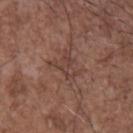Case summary:
* workup — total-body-photography surveillance lesion; no biopsy
* image — ~15 mm crop, total-body skin-cancer survey
* patient — male, aged 68–72
* TBP lesion metrics — a footprint of about 5.5 mm² and two-axis asymmetry of about 0.6; a color-variation rating of about 4.5/10 and radial color variation of about 1.5; a detector confidence of about 80 out of 100 that the crop contains a lesion
* location — the chest
* lesion size — about 3.5 mm